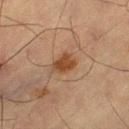Findings:
– notes — imaged on a skin check; not biopsied
– patient — male, about 65 years old
– image — 15 mm crop, total-body photography
– automated metrics — a lesion color around L≈36 a*≈17 b*≈28 in CIELAB, a lesion–skin lightness drop of about 9, and a lesion-to-skin contrast of about 8.5 (normalized; higher = more distinct); an automated nevus-likeness rating near 95 out of 100 and a detector confidence of about 100 out of 100 that the crop contains a lesion
– location — the right thigh
– size — ≈3 mm